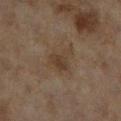follow-up: catalogued during a skin exam; not biopsied
lesion size: about 3.5 mm
subject: female, aged approximately 60
body site: the right lower leg
acquisition: ~15 mm crop, total-body skin-cancer survey
automated lesion analysis: a lesion area of about 6 mm² and an eccentricity of roughly 0.55; a detector confidence of about 100 out of 100 that the crop contains a lesion